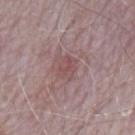follow-up: total-body-photography surveillance lesion; no biopsy
patient: male, aged 63–67
anatomic site: the mid back
acquisition: ~15 mm tile from a whole-body skin photo
tile lighting: white-light